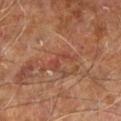notes = no biopsy performed (imaged during a skin exam) | subject = male, aged around 60 | automated metrics = a footprint of about 4.5 mm² and a shape eccentricity near 0.9; an average lesion color of about L≈43 a*≈26 b*≈29 (CIELAB), roughly 6 lightness units darker than nearby skin, and a normalized border contrast of about 5; a within-lesion color-variation index near 1/10 and a peripheral color-asymmetry measure near 0.5; an automated nevus-likeness rating near 0 out of 100 and a lesion-detection confidence of about 85/100 | acquisition = 15 mm crop, total-body photography | size = about 3.5 mm | lighting = cross-polarized illumination | body site = the right leg.